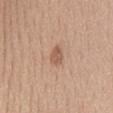No biopsy was performed on this lesion — it was imaged during a full skin examination and was not determined to be concerning. Measured at roughly 3 mm in maximum diameter. A lesion tile, about 15 mm wide, cut from a 3D total-body photograph. The lesion is on the mid back. This is a white-light tile. A female patient, aged 53 to 57.